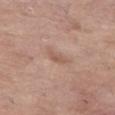Clinical impression:
The lesion was photographed on a routine skin check and not biopsied; there is no pathology result.
Acquisition and patient details:
Captured under white-light illumination. From the left thigh. This image is a 15 mm lesion crop taken from a total-body photograph. A female subject, approximately 65 years of age. Longest diameter approximately 2.5 mm. Automated image analysis of the tile measured a mean CIELAB color near L≈56 a*≈19 b*≈28, roughly 8 lightness units darker than nearby skin, and a normalized border contrast of about 6.